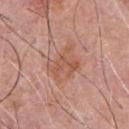Impression:
Imaged during a routine full-body skin examination; the lesion was not biopsied and no histopathology is available.
Context:
The patient is a male about 60 years old. Located on the chest. Captured under white-light illumination. Cropped from a total-body skin-imaging series; the visible field is about 15 mm. Approximately 4 mm at its widest.